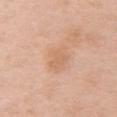The lesion was photographed on a routine skin check and not biopsied; there is no pathology result.
A female patient, about 50 years old.
The lesion is on the chest.
The recorded lesion diameter is about 3.5 mm.
A lesion tile, about 15 mm wide, cut from a 3D total-body photograph.
The tile uses white-light illumination.